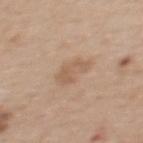Captured during whole-body skin photography for melanoma surveillance; the lesion was not biopsied. On the back. The recorded lesion diameter is about 3.5 mm. An algorithmic analysis of the crop reported an area of roughly 5.5 mm², a shape eccentricity near 0.8, and two-axis asymmetry of about 0.45. The analysis additionally found internal color variation of about 1 on a 0–10 scale and peripheral color asymmetry of about 0.5. A female subject aged approximately 65. The tile uses white-light illumination. A close-up tile cropped from a whole-body skin photograph, about 15 mm across.